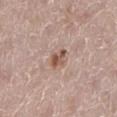Clinical impression:
Imaged during a routine full-body skin examination; the lesion was not biopsied and no histopathology is available.
Context:
Automated tile analysis of the lesion measured a border-irregularity index near 2.5/10, internal color variation of about 4.5 on a 0–10 scale, and a peripheral color-asymmetry measure near 1. And it measured a detector confidence of about 100 out of 100 that the crop contains a lesion. This image is a 15 mm lesion crop taken from a total-body photograph. On the left lower leg. The tile uses white-light illumination. A female patient, aged around 50. Approximately 2.5 mm at its widest.